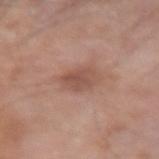biopsy status: no biopsy performed (imaged during a skin exam)
subject: male, about 80 years old
automated metrics: a lesion area of about 6 mm², an outline eccentricity of about 0.6 (0 = round, 1 = elongated), and two-axis asymmetry of about 0.25; internal color variation of about 2.5 on a 0–10 scale and radial color variation of about 1
size: ≈3 mm
body site: the right forearm
illumination: white-light
imaging modality: ~15 mm crop, total-body skin-cancer survey